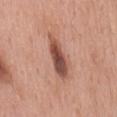lesion size: about 6 mm
patient: male, aged 58 to 62
location: the mid back
lighting: white-light illumination
TBP lesion metrics: an area of roughly 10 mm², an eccentricity of roughly 0.95, and a symmetry-axis asymmetry near 0.25; about 15 CIELAB-L* units darker than the surrounding skin and a normalized border contrast of about 10; an automated nevus-likeness rating near 90 out of 100 and lesion-presence confidence of about 100/100
image: ~15 mm crop, total-body skin-cancer survey